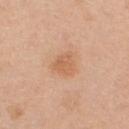Findings:
* workup — total-body-photography surveillance lesion; no biopsy
* imaging modality — ~15 mm crop, total-body skin-cancer survey
* patient — male, approximately 50 years of age
* lesion diameter — ≈3 mm
* site — the chest
* TBP lesion metrics — an eccentricity of roughly 0.35 and two-axis asymmetry of about 0.35; a detector confidence of about 100 out of 100 that the crop contains a lesion
* tile lighting — white-light